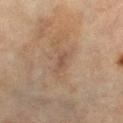Assessment: Recorded during total-body skin imaging; not selected for excision or biopsy. Background: This is a cross-polarized tile. A female subject, in their 70s. Automated image analysis of the tile measured an area of roughly 3 mm² and a shape eccentricity near 0.9. The software also gave lesion-presence confidence of about 100/100. A close-up tile cropped from a whole-body skin photograph, about 15 mm across. The lesion is on the right lower leg. Longest diameter approximately 3 mm.